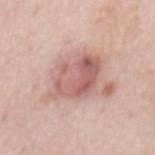Captured during whole-body skin photography for melanoma surveillance; the lesion was not biopsied.
The lesion is located on the mid back.
Approximately 5 mm at its widest.
A 15 mm crop from a total-body photograph taken for skin-cancer surveillance.
A male subject, in their 40s.
Automated image analysis of the tile measured a footprint of about 16 mm². The analysis additionally found a within-lesion color-variation index near 7/10 and a peripheral color-asymmetry measure near 2.5.